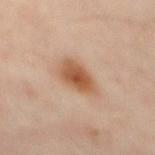– follow-up: imaged on a skin check; not biopsied